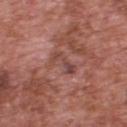  biopsy_status: not biopsied; imaged during a skin examination
  automated_metrics:
    area_mm2_approx: 4.5
    eccentricity: 0.9
    shape_asymmetry: 0.5
    border_irregularity_0_10: 6.5
    color_variation_0_10: 0.0
    peripheral_color_asymmetry: 0.0
    nevus_likeness_0_100: 0
    lesion_detection_confidence_0_100: 65
  patient:
    sex: male
    age_approx: 70
  lesion_size:
    long_diameter_mm_approx: 3.5
  image:
    source: total-body photography crop
    field_of_view_mm: 15
  site: upper back